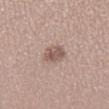Part of a total-body skin-imaging series; this lesion was reviewed on a skin check and was not flagged for biopsy. From the right lower leg. Approximately 3 mm at its widest. The subject is a female roughly 40 years of age. A lesion tile, about 15 mm wide, cut from a 3D total-body photograph. The tile uses white-light illumination. Automated tile analysis of the lesion measured a lesion area of about 6 mm², a shape eccentricity near 0.4, and a shape-asymmetry score of about 0.2 (0 = symmetric). And it measured a color-variation rating of about 3.5/10 and peripheral color asymmetry of about 1. And it measured a lesion-detection confidence of about 100/100.